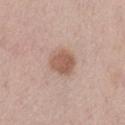Q: Was this lesion biopsied?
A: imaged on a skin check; not biopsied
Q: How large is the lesion?
A: ~3.5 mm (longest diameter)
Q: Lesion location?
A: the left thigh
Q: What kind of image is this?
A: 15 mm crop, total-body photography
Q: What did automated image analysis measure?
A: a border-irregularity index near 1.5/10, a within-lesion color-variation index near 2.5/10, and a peripheral color-asymmetry measure near 1
Q: Patient demographics?
A: male, aged around 75
Q: How was the tile lit?
A: white-light